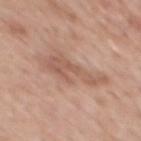The lesion was tiled from a total-body skin photograph and was not biopsied. On the mid back. Longest diameter approximately 6 mm. This image is a 15 mm lesion crop taken from a total-body photograph. A male subject, aged 58–62. This is a white-light tile. Automated tile analysis of the lesion measured a lesion color around L≈57 a*≈20 b*≈28 in CIELAB, roughly 8 lightness units darker than nearby skin, and a normalized lesion–skin contrast near 5.5. The analysis additionally found a border-irregularity rating of about 8/10 and peripheral color asymmetry of about 1. And it measured lesion-presence confidence of about 100/100.